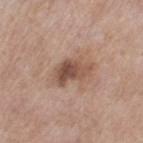workup: catalogued during a skin exam; not biopsied
image-analysis metrics: a lesion color around L≈51 a*≈19 b*≈27 in CIELAB and a normalized lesion–skin contrast near 8.5; a border-irregularity rating of about 4/10 and peripheral color asymmetry of about 2.5; a classifier nevus-likeness of about 15/100 and a detector confidence of about 100 out of 100 that the crop contains a lesion
image: ~15 mm crop, total-body skin-cancer survey
diameter: ≈5 mm
body site: the left thigh
lighting: white-light
patient: female, approximately 55 years of age